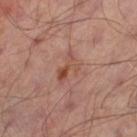notes = no biopsy performed (imaged during a skin exam); body site = the left thigh; imaging modality = ~15 mm crop, total-body skin-cancer survey; patient = male, approximately 65 years of age.